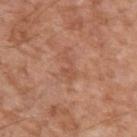No biopsy was performed on this lesion — it was imaged during a full skin examination and was not determined to be concerning.
Approximately 3 mm at its widest.
On the left upper arm.
The subject is a male aged around 55.
Cropped from a whole-body photographic skin survey; the tile spans about 15 mm.
The lesion-visualizer software estimated a lesion area of about 3 mm² and a shape eccentricity near 0.9. The analysis additionally found a border-irregularity rating of about 7.5/10, a color-variation rating of about 0/10, and radial color variation of about 0. And it measured lesion-presence confidence of about 100/100.
This is a white-light tile.